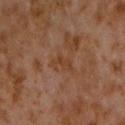Q: Was a biopsy performed?
A: total-body-photography surveillance lesion; no biopsy
Q: What is the anatomic site?
A: the chest
Q: What lighting was used for the tile?
A: cross-polarized
Q: What kind of image is this?
A: 15 mm crop, total-body photography
Q: What is the lesion's diameter?
A: ~3 mm (longest diameter)
Q: Patient demographics?
A: male, aged 58 to 62